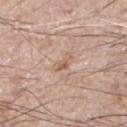Imaged during a routine full-body skin examination; the lesion was not biopsied and no histopathology is available. The tile uses white-light illumination. On the front of the torso. The patient is a male roughly 75 years of age. Cropped from a whole-body photographic skin survey; the tile spans about 15 mm. Longest diameter approximately 3 mm.